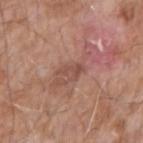Impression: Captured during whole-body skin photography for melanoma surveillance; the lesion was not biopsied. Image and clinical context: The lesion is on the arm. A male patient, in their mid- to late 70s. This image is a 15 mm lesion crop taken from a total-body photograph. Captured under white-light illumination. Automated tile analysis of the lesion measured an average lesion color of about L≈50 a*≈22 b*≈26 (CIELAB), roughly 8 lightness units darker than nearby skin, and a lesion-to-skin contrast of about 5.5 (normalized; higher = more distinct). And it measured a border-irregularity index near 3.5/10 and peripheral color asymmetry of about 1. The software also gave a classifier nevus-likeness of about 0/100 and a lesion-detection confidence of about 100/100.